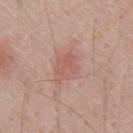notes — no biopsy performed (imaged during a skin exam); diameter — ~3 mm (longest diameter); site — the front of the torso; subject — male, approximately 70 years of age; illumination — white-light illumination; image — ~15 mm crop, total-body skin-cancer survey; TBP lesion metrics — a border-irregularity index near 2.5/10, a color-variation rating of about 2/10, and a peripheral color-asymmetry measure near 1.